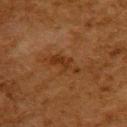Case summary:
* follow-up · catalogued during a skin exam; not biopsied
* image · 15 mm crop, total-body photography
* subject · female, aged 48 to 52
* location · the upper back
* illumination · cross-polarized